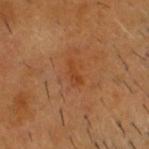Recorded during total-body skin imaging; not selected for excision or biopsy.